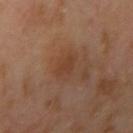Clinical impression:
Recorded during total-body skin imaging; not selected for excision or biopsy.
Context:
About 2.5 mm across. A 15 mm close-up extracted from a 3D total-body photography capture. The patient is a female aged around 55. The lesion is located on the right upper arm.